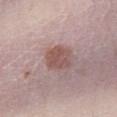Recorded during total-body skin imaging; not selected for excision or biopsy.